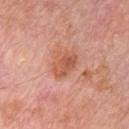Q: Is there a histopathology result?
A: imaged on a skin check; not biopsied
Q: Who is the patient?
A: male, approximately 65 years of age
Q: What is the anatomic site?
A: the chest
Q: How was this image acquired?
A: total-body-photography crop, ~15 mm field of view